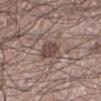Notes:
– biopsy status: catalogued during a skin exam; not biopsied
– image source: ~15 mm tile from a whole-body skin photo
– body site: the left lower leg
– subject: male, aged around 60